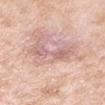follow-up = total-body-photography surveillance lesion; no biopsy
size = ≈5 mm
location = the left upper arm
tile lighting = white-light
patient = male, about 55 years old
image = ~15 mm crop, total-body skin-cancer survey